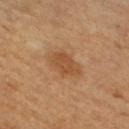| field | value |
|---|---|
| notes | no biopsy performed (imaged during a skin exam) |
| tile lighting | cross-polarized |
| size | ≈4 mm |
| acquisition | ~15 mm tile from a whole-body skin photo |
| site | the right upper arm |
| subject | female, roughly 60 years of age |